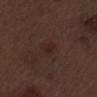Assessment:
Captured during whole-body skin photography for melanoma surveillance; the lesion was not biopsied.
Acquisition and patient details:
Captured under white-light illumination. Measured at roughly 2.5 mm in maximum diameter. The lesion is located on the left upper arm. A male subject, about 70 years old. Cropped from a total-body skin-imaging series; the visible field is about 15 mm. Automated image analysis of the tile measured a lesion area of about 3 mm² and two-axis asymmetry of about 0.25. It also reported a color-variation rating of about 1/10 and radial color variation of about 0.5. The analysis additionally found a nevus-likeness score of about 20/100.